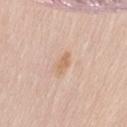Q: Was this lesion biopsied?
A: total-body-photography surveillance lesion; no biopsy
Q: What is the lesion's diameter?
A: ~2.5 mm (longest diameter)
Q: How was this image acquired?
A: 15 mm crop, total-body photography
Q: What lighting was used for the tile?
A: white-light
Q: Where on the body is the lesion?
A: the lower back
Q: What are the patient's age and sex?
A: male, in their mid- to late 80s or older
Q: What did automated image analysis measure?
A: an average lesion color of about L≈66 a*≈18 b*≈34 (CIELAB) and a normalized border contrast of about 6.5; an automated nevus-likeness rating near 5 out of 100 and a lesion-detection confidence of about 100/100